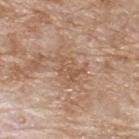The lesion was tiled from a total-body skin photograph and was not biopsied. The recorded lesion diameter is about 3 mm. This image is a 15 mm lesion crop taken from a total-body photograph. An algorithmic analysis of the crop reported a border-irregularity rating of about 5.5/10, a within-lesion color-variation index near 2/10, and a peripheral color-asymmetry measure near 1. The analysis additionally found a detector confidence of about 100 out of 100 that the crop contains a lesion. The lesion is located on the upper back. A male patient, about 80 years old.